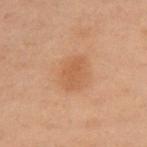Assessment:
Recorded during total-body skin imaging; not selected for excision or biopsy.
Clinical summary:
The lesion is located on the chest. A 15 mm close-up tile from a total-body photography series done for melanoma screening. The patient is a female in their mid- to late 50s. The total-body-photography lesion software estimated a mean CIELAB color near L≈47 a*≈20 b*≈31 and a lesion–skin lightness drop of about 6. The software also gave lesion-presence confidence of about 100/100. Captured under cross-polarized illumination.